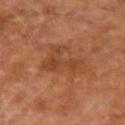<lesion>
<biopsy_status>not biopsied; imaged during a skin examination</biopsy_status>
<lighting>cross-polarized</lighting>
<lesion_size>
  <long_diameter_mm_approx>5.5</long_diameter_mm_approx>
</lesion_size>
<patient>
  <sex>male</sex>
  <age_approx>55</age_approx>
</patient>
<site>right forearm</site>
<image>
  <source>total-body photography crop</source>
  <field_of_view_mm>15</field_of_view_mm>
</image>
</lesion>